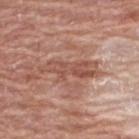follow-up — total-body-photography surveillance lesion; no biopsy | patient — female, in their 60s | size — about 6.5 mm | automated metrics — an area of roughly 10 mm², an eccentricity of roughly 0.95, and a symmetry-axis asymmetry near 0.45; a normalized border contrast of about 6.5; a color-variation rating of about 3.5/10 and radial color variation of about 1.5; an automated nevus-likeness rating near 0 out of 100 and a lesion-detection confidence of about 65/100 | imaging modality — ~15 mm tile from a whole-body skin photo | location — the back.